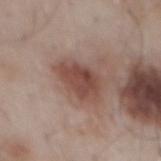Case summary:
– biopsy status — total-body-photography surveillance lesion; no biopsy
– image source — 15 mm crop, total-body photography
– lighting — white-light
– subject — male, aged 53 to 57
– body site — the mid back